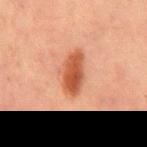Recorded during total-body skin imaging; not selected for excision or biopsy. Cropped from a whole-body photographic skin survey; the tile spans about 15 mm. The subject is a male aged approximately 65. Located on the front of the torso.